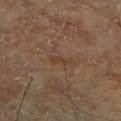| key | value |
|---|---|
| workup | total-body-photography surveillance lesion; no biopsy |
| illumination | cross-polarized |
| imaging modality | ~15 mm tile from a whole-body skin photo |
| patient | male, aged 68–72 |
| site | the right lower leg |
| size | about 2.5 mm |